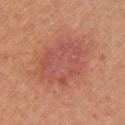workup: imaged on a skin check; not biopsied
imaging modality: ~15 mm crop, total-body skin-cancer survey
automated lesion analysis: a footprint of about 27 mm² and a shape-asymmetry score of about 0.15 (0 = symmetric)
patient: female, about 40 years old
lighting: white-light illumination
anatomic site: the right upper arm
lesion size: ~7.5 mm (longest diameter)